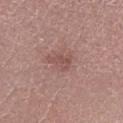{"biopsy_status": "not biopsied; imaged during a skin examination", "patient": {"sex": "male", "age_approx": 20}, "site": "leg", "lighting": "white-light", "image": {"source": "total-body photography crop", "field_of_view_mm": 15}, "lesion_size": {"long_diameter_mm_approx": 3.0}, "automated_metrics": {"vs_skin_darker_L": 7.0, "vs_skin_contrast_norm": 5.5, "border_irregularity_0_10": 5.5, "color_variation_0_10": 2.0, "nevus_likeness_0_100": 0, "lesion_detection_confidence_0_100": 100}}